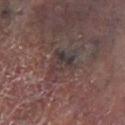Image and clinical context:
On the left lower leg. A roughly 15 mm field-of-view crop from a total-body skin photograph. A male patient in their mid- to late 60s. The total-body-photography lesion software estimated a detector confidence of about 55 out of 100 that the crop contains a lesion. About 4.5 mm across. This is a cross-polarized tile.